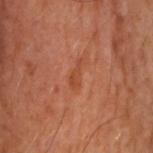  biopsy_status: not biopsied; imaged during a skin examination
  lesion_size:
    long_diameter_mm_approx: 3.0
  patient:
    sex: male
    age_approx: 50
  automated_metrics:
    eccentricity: 0.95
    shape_asymmetry: 0.5
    border_irregularity_0_10: 5.5
    color_variation_0_10: 0.0
    peripheral_color_asymmetry: 0.0
    nevus_likeness_0_100: 0
    lesion_detection_confidence_0_100: 100
  site: upper back
  image:
    source: total-body photography crop
    field_of_view_mm: 15
  lighting: cross-polarized